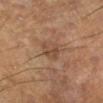{"image": {"source": "total-body photography crop", "field_of_view_mm": 15}, "lighting": "cross-polarized", "patient": {"sex": "male", "age_approx": 60}, "site": "left lower leg", "automated_metrics": {"area_mm2_approx": 4.5, "eccentricity": 0.65, "shape_asymmetry": 0.4, "cielab_L": 44, "cielab_a": 17, "cielab_b": 29, "vs_skin_contrast_norm": 5.5, "peripheral_color_asymmetry": 1.0, "nevus_likeness_0_100": 0, "lesion_detection_confidence_0_100": 100}, "lesion_size": {"long_diameter_mm_approx": 2.5}}